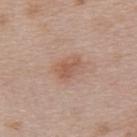biopsy status = no biopsy performed (imaged during a skin exam)
automated lesion analysis = an automated nevus-likeness rating near 40 out of 100 and lesion-presence confidence of about 100/100
anatomic site = the upper back
image source = ~15 mm crop, total-body skin-cancer survey
lighting = white-light illumination
size = ~3.5 mm (longest diameter)
patient = female, in their 50s